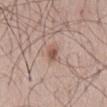Clinical impression: Recorded during total-body skin imaging; not selected for excision or biopsy. Acquisition and patient details: Automated image analysis of the tile measured an area of roughly 3.5 mm², an eccentricity of roughly 0.85, and two-axis asymmetry of about 0.2. It also reported a lesion color around L≈55 a*≈20 b*≈25 in CIELAB, roughly 10 lightness units darker than nearby skin, and a normalized border contrast of about 7.5. It also reported a classifier nevus-likeness of about 90/100. About 2.5 mm across. A male patient roughly 50 years of age. This is a white-light tile. A 15 mm crop from a total-body photograph taken for skin-cancer surveillance. From the mid back.